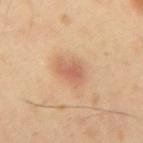lighting: cross-polarized
patient:
  sex: male
  age_approx: 40
image:
  source: total-body photography crop
  field_of_view_mm: 15
site: right upper arm
lesion_size:
  long_diameter_mm_approx: 3.5